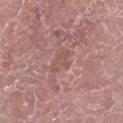Part of a total-body skin-imaging series; this lesion was reviewed on a skin check and was not flagged for biopsy.